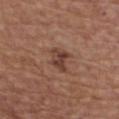notes — total-body-photography surveillance lesion; no biopsy | patient — male, aged around 65 | automated lesion analysis — a footprint of about 5 mm², a shape eccentricity near 0.7, and a symmetry-axis asymmetry near 0.3; a border-irregularity rating of about 3.5/10, a within-lesion color-variation index near 4/10, and a peripheral color-asymmetry measure near 1.5; an automated nevus-likeness rating near 10 out of 100 and a lesion-detection confidence of about 100/100 | lighting — white-light illumination | site — the chest | lesion size — about 3 mm | imaging modality — total-body-photography crop, ~15 mm field of view.